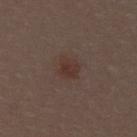Q: Was a biopsy performed?
A: imaged on a skin check; not biopsied
Q: Lesion size?
A: ≈3 mm
Q: What are the patient's age and sex?
A: female, aged 28–32
Q: What is the anatomic site?
A: the upper back
Q: How was this image acquired?
A: ~15 mm tile from a whole-body skin photo
Q: Illumination type?
A: white-light